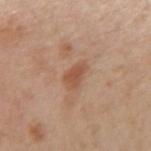Clinical impression:
Imaged during a routine full-body skin examination; the lesion was not biopsied and no histopathology is available.
Background:
From the arm. A 15 mm close-up tile from a total-body photography series done for melanoma screening. A female subject approximately 40 years of age. The lesion's longest dimension is about 3 mm. The total-body-photography lesion software estimated a lesion-to-skin contrast of about 7 (normalized; higher = more distinct). It also reported a detector confidence of about 100 out of 100 that the crop contains a lesion. The tile uses white-light illumination.